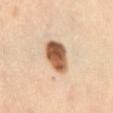Image and clinical context:
Automated image analysis of the tile measured an eccentricity of roughly 0.75 and a symmetry-axis asymmetry near 0.2. And it measured border irregularity of about 1.5 on a 0–10 scale, a color-variation rating of about 7.5/10, and a peripheral color-asymmetry measure near 3. And it measured an automated nevus-likeness rating near 100 out of 100 and a lesion-detection confidence of about 100/100. A 15 mm crop from a total-body photograph taken for skin-cancer surveillance. A female subject about 55 years old. The lesion is located on the abdomen.
Diagnosis:
The lesion was biopsied, and histopathology showed an indeterminate (borderline) lesion: atypical melanocytic neoplasm.